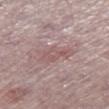No biopsy was performed on this lesion — it was imaged during a full skin examination and was not determined to be concerning.
Measured at roughly 4 mm in maximum diameter.
This is a white-light tile.
Automated image analysis of the tile measured an average lesion color of about L≈55 a*≈19 b*≈19 (CIELAB) and about 7 CIELAB-L* units darker than the surrounding skin. The software also gave a color-variation rating of about 2/10 and a peripheral color-asymmetry measure near 0.5. The software also gave an automated nevus-likeness rating near 0 out of 100 and lesion-presence confidence of about 75/100.
A male subject in their mid- to late 70s.
Cropped from a total-body skin-imaging series; the visible field is about 15 mm.
From the right thigh.